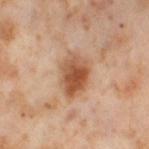{
  "biopsy_status": "not biopsied; imaged during a skin examination",
  "lighting": "cross-polarized",
  "automated_metrics": {
    "cielab_L": 55,
    "cielab_a": 23,
    "cielab_b": 35,
    "vs_skin_darker_L": 13.0,
    "vs_skin_contrast_norm": 9.0
  },
  "lesion_size": {
    "long_diameter_mm_approx": 5.0
  },
  "image": {
    "source": "total-body photography crop",
    "field_of_view_mm": 15
  },
  "patient": {
    "sex": "female",
    "age_approx": 55
  },
  "site": "leg"
}